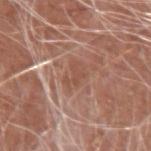Case summary:
* follow-up — no biopsy performed (imaged during a skin exam)
* image source — ~15 mm crop, total-body skin-cancer survey
* lighting — white-light illumination
* subject — male, aged around 80
* lesion diameter — ≈3 mm
* site — the arm